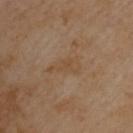Imaged during a routine full-body skin examination; the lesion was not biopsied and no histopathology is available.